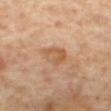Q: Was a biopsy performed?
A: imaged on a skin check; not biopsied
Q: What are the patient's age and sex?
A: male, aged around 65
Q: What is the anatomic site?
A: the mid back
Q: What lighting was used for the tile?
A: cross-polarized
Q: How was this image acquired?
A: ~15 mm crop, total-body skin-cancer survey